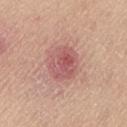biopsy status — total-body-photography surveillance lesion; no biopsy | acquisition — ~15 mm crop, total-body skin-cancer survey | tile lighting — white-light | subject — female, aged around 40 | size — about 4 mm | body site — the left thigh.